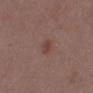workup: catalogued during a skin exam; not biopsied | site: the back | subject: male, about 50 years old | acquisition: ~15 mm crop, total-body skin-cancer survey.